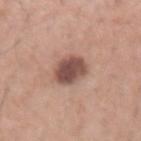Recorded during total-body skin imaging; not selected for excision or biopsy. About 4 mm across. From the right forearm. A male patient aged 38 to 42. A roughly 15 mm field-of-view crop from a total-body skin photograph.